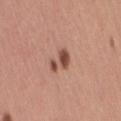Impression: No biopsy was performed on this lesion — it was imaged during a full skin examination and was not determined to be concerning. Acquisition and patient details: An algorithmic analysis of the crop reported a footprint of about 5 mm², a shape eccentricity near 0.8, and a shape-asymmetry score of about 0.55 (0 = symmetric). It also reported a color-variation rating of about 2.5/10 and peripheral color asymmetry of about 1. A female subject roughly 30 years of age. The tile uses white-light illumination. About 3 mm across. The lesion is on the leg. A 15 mm close-up tile from a total-body photography series done for melanoma screening.